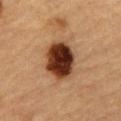| field | value |
|---|---|
| workup | imaged on a skin check; not biopsied |
| subject | male, about 85 years old or older |
| location | the abdomen |
| image | ~15 mm tile from a whole-body skin photo |